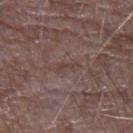Findings:
* automated metrics · an area of roughly 2 mm², an outline eccentricity of about 0.95 (0 = round, 1 = elongated), and two-axis asymmetry of about 0.4; a lesion color around L≈40 a*≈16 b*≈20 in CIELAB and about 6 CIELAB-L* units darker than the surrounding skin
* body site · the left forearm
* acquisition · total-body-photography crop, ~15 mm field of view
* subject · male, aged approximately 65
* diameter · about 3 mm
* illumination · white-light